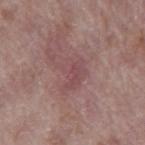Clinical impression:
The lesion was photographed on a routine skin check and not biopsied; there is no pathology result.
Clinical summary:
Approximately 3.5 mm at its widest. A male subject roughly 70 years of age. This is a white-light tile. This image is a 15 mm lesion crop taken from a total-body photograph. From the mid back.